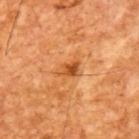The lesion was tiled from a total-body skin photograph and was not biopsied. About 3 mm across. A male subject aged approximately 65. A lesion tile, about 15 mm wide, cut from a 3D total-body photograph. Imaged with cross-polarized lighting.